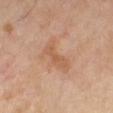Clinical impression:
Imaged during a routine full-body skin examination; the lesion was not biopsied and no histopathology is available.
Context:
A female subject aged approximately 70. A 15 mm close-up tile from a total-body photography series done for melanoma screening. On the right lower leg.